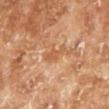Case summary:
– workup — imaged on a skin check; not biopsied
– image source — 15 mm crop, total-body photography
– subject — male, approximately 70 years of age
– illumination — cross-polarized
– automated lesion analysis — a lesion area of about 4 mm² and an outline eccentricity of about 0.85 (0 = round, 1 = elongated); a within-lesion color-variation index near 1/10
– site — the left lower leg
– size — about 3 mm